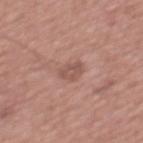<record>
  <biopsy_status>not biopsied; imaged during a skin examination</biopsy_status>
  <lesion_size>
    <long_diameter_mm_approx>2.5</long_diameter_mm_approx>
  </lesion_size>
  <patient>
    <sex>male</sex>
    <age_approx>45</age_approx>
  </patient>
  <automated_metrics>
    <area_mm2_approx>4.0</area_mm2_approx>
    <eccentricity>0.6</eccentricity>
    <shape_asymmetry>0.25</shape_asymmetry>
    <cielab_L>53</cielab_L>
    <cielab_a>21</cielab_a>
    <cielab_b>25</cielab_b>
    <vs_skin_darker_L>8.0</vs_skin_darker_L>
    <vs_skin_contrast_norm>6.0</vs_skin_contrast_norm>
  </automated_metrics>
  <image>
    <source>total-body photography crop</source>
    <field_of_view_mm>15</field_of_view_mm>
  </image>
  <site>front of the torso</site>
</record>